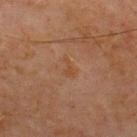Clinical impression:
Recorded during total-body skin imaging; not selected for excision or biopsy.
Background:
This is a cross-polarized tile. A roughly 15 mm field-of-view crop from a total-body skin photograph. The patient is a male aged around 70. Automated tile analysis of the lesion measured a shape eccentricity near 0.85. The analysis additionally found a border-irregularity index near 5.5/10, internal color variation of about 0 on a 0–10 scale, and a peripheral color-asymmetry measure near 0. The lesion is on the chest.